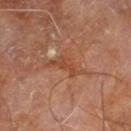Recorded during total-body skin imaging; not selected for excision or biopsy. A male patient approximately 60 years of age. On the right leg. Captured under cross-polarized illumination. Longest diameter approximately 5 mm. A region of skin cropped from a whole-body photographic capture, roughly 15 mm wide.